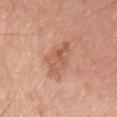The tile uses white-light illumination. The lesion is on the chest. The patient is a male in their mid- to late 70s. Approximately 4.5 mm at its widest. A 15 mm close-up extracted from a 3D total-body photography capture.